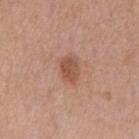<lesion>
<site>mid back</site>
<patient>
  <sex>male</sex>
  <age_approx>70</age_approx>
</patient>
<automated_metrics>
  <cielab_L>52</cielab_L>
  <cielab_a>23</cielab_a>
  <cielab_b>30</cielab_b>
  <vs_skin_contrast_norm>7.5</vs_skin_contrast_norm>
  <nevus_likeness_0_100>65</nevus_likeness_0_100>
  <lesion_detection_confidence_0_100>100</lesion_detection_confidence_0_100>
</automated_metrics>
<image>
  <source>total-body photography crop</source>
  <field_of_view_mm>15</field_of_view_mm>
</image>
<lesion_size>
  <long_diameter_mm_approx>3.0</long_diameter_mm_approx>
</lesion_size>
<lighting>white-light</lighting>
</lesion>